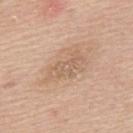biopsy_status: not biopsied; imaged during a skin examination
patient:
  sex: male
  age_approx: 50
lesion_size:
  long_diameter_mm_approx: 4.5
image:
  source: total-body photography crop
  field_of_view_mm: 15
site: mid back